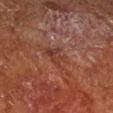imaging modality: ~15 mm tile from a whole-body skin photo
lesion size: about 3 mm
anatomic site: the right lower leg
subject: male, aged around 65
lighting: cross-polarized
TBP lesion metrics: a border-irregularity rating of about 3.5/10, a color-variation rating of about 4.5/10, and a peripheral color-asymmetry measure near 1.5